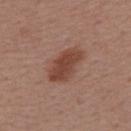Captured during whole-body skin photography for melanoma surveillance; the lesion was not biopsied. A female subject roughly 55 years of age. The total-body-photography lesion software estimated a mean CIELAB color near L≈44 a*≈22 b*≈27, a lesion–skin lightness drop of about 10, and a normalized lesion–skin contrast near 8.5. It also reported internal color variation of about 2.5 on a 0–10 scale. It also reported a classifier nevus-likeness of about 95/100 and a detector confidence of about 100 out of 100 that the crop contains a lesion. The lesion is located on the right upper arm. The recorded lesion diameter is about 5 mm. A region of skin cropped from a whole-body photographic capture, roughly 15 mm wide.